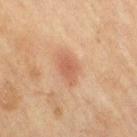Impression:
The lesion was tiled from a total-body skin photograph and was not biopsied.
Clinical summary:
A 15 mm close-up extracted from a 3D total-body photography capture. Measured at roughly 3.5 mm in maximum diameter. Imaged with cross-polarized lighting. The lesion is on the right thigh. A female patient, aged 58 to 62.